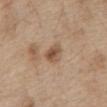image:
  source: total-body photography crop
  field_of_view_mm: 15
patient:
  sex: male
  age_approx: 70
site: front of the torso